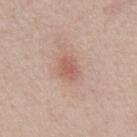| key | value |
|---|---|
| biopsy status | imaged on a skin check; not biopsied |
| anatomic site | the chest |
| image | ~15 mm tile from a whole-body skin photo |
| automated lesion analysis | an area of roughly 4.5 mm², an eccentricity of roughly 0.65, and a shape-asymmetry score of about 0.25 (0 = symmetric); a mean CIELAB color near L≈58 a*≈23 b*≈27, roughly 9 lightness units darker than nearby skin, and a normalized border contrast of about 6 |
| diameter | about 2.5 mm |
| patient | male, aged around 55 |
| tile lighting | white-light illumination |